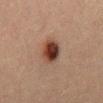| field | value |
|---|---|
| workup | no biopsy performed (imaged during a skin exam) |
| tile lighting | cross-polarized |
| patient | male, roughly 50 years of age |
| image | total-body-photography crop, ~15 mm field of view |
| site | the abdomen |